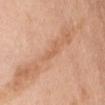Assessment:
Part of a total-body skin-imaging series; this lesion was reviewed on a skin check and was not flagged for biopsy.
Context:
The subject is a female about 65 years old. An algorithmic analysis of the crop reported an outline eccentricity of about 0.95 (0 = round, 1 = elongated). And it measured a border-irregularity rating of about 5.5/10, a color-variation rating of about 0/10, and radial color variation of about 0. Located on the abdomen. Approximately 3 mm at its widest. Imaged with white-light lighting. A lesion tile, about 15 mm wide, cut from a 3D total-body photograph.